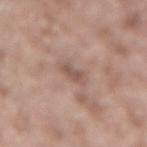Q: What are the patient's age and sex?
A: male, in their mid- to late 50s
Q: Where on the body is the lesion?
A: the lower back
Q: What is the lesion's diameter?
A: ≈2.5 mm
Q: Illumination type?
A: white-light illumination
Q: How was this image acquired?
A: ~15 mm tile from a whole-body skin photo